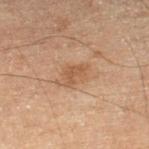No biopsy was performed on this lesion — it was imaged during a full skin examination and was not determined to be concerning. Approximately 3 mm at its widest. Automated image analysis of the tile measured a lesion area of about 5 mm², a shape eccentricity near 0.7, and two-axis asymmetry of about 0.4. From the left lower leg. A male patient approximately 70 years of age. A lesion tile, about 15 mm wide, cut from a 3D total-body photograph.